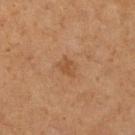Notes:
- workup — total-body-photography surveillance lesion; no biopsy